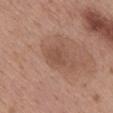Q: Was this lesion biopsied?
A: catalogued during a skin exam; not biopsied
Q: Who is the patient?
A: female, approximately 50 years of age
Q: What is the lesion's diameter?
A: ~2.5 mm (longest diameter)
Q: What is the anatomic site?
A: the upper back
Q: Illumination type?
A: white-light
Q: What is the imaging modality?
A: 15 mm crop, total-body photography
Q: What did automated image analysis measure?
A: a lesion area of about 4 mm², an outline eccentricity of about 0.75 (0 = round, 1 = elongated), and a shape-asymmetry score of about 0.25 (0 = symmetric); border irregularity of about 2 on a 0–10 scale, a color-variation rating of about 1/10, and peripheral color asymmetry of about 0.5; a nevus-likeness score of about 10/100